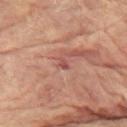{"biopsy_status": "not biopsied; imaged during a skin examination", "lighting": "cross-polarized", "site": "left arm", "image": {"source": "total-body photography crop", "field_of_view_mm": 15}, "patient": {"sex": "female", "age_approx": 80}}